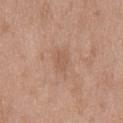The patient is a male aged around 50. Located on the chest. A close-up tile cropped from a whole-body skin photograph, about 15 mm across. The tile uses white-light illumination.